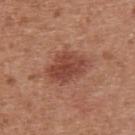Impression: Part of a total-body skin-imaging series; this lesion was reviewed on a skin check and was not flagged for biopsy. Acquisition and patient details: Longest diameter approximately 5 mm. From the upper back. Automated image analysis of the tile measured a footprint of about 12 mm² and a symmetry-axis asymmetry near 0.2. And it measured a lesion color around L≈45 a*≈26 b*≈29 in CIELAB, roughly 10 lightness units darker than nearby skin, and a lesion-to-skin contrast of about 8 (normalized; higher = more distinct). And it measured a border-irregularity index near 2.5/10 and a within-lesion color-variation index near 3.5/10. The analysis additionally found a nevus-likeness score of about 70/100 and a lesion-detection confidence of about 100/100. A roughly 15 mm field-of-view crop from a total-body skin photograph. A male subject aged 63 to 67. The tile uses white-light illumination.